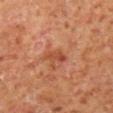This lesion was catalogued during total-body skin photography and was not selected for biopsy. The lesion is located on the right lower leg. A male subject, aged 58–62. A close-up tile cropped from a whole-body skin photograph, about 15 mm across.